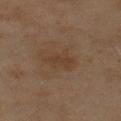<tbp_lesion>
<biopsy_status>not biopsied; imaged during a skin examination</biopsy_status>
</tbp_lesion>